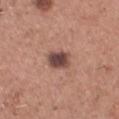This lesion was catalogued during total-body skin photography and was not selected for biopsy.
Located on the chest.
A male patient about 45 years old.
The total-body-photography lesion software estimated an area of roughly 6 mm², an eccentricity of roughly 0.7, and two-axis asymmetry of about 0.2. The analysis additionally found a lesion color around L≈45 a*≈20 b*≈22 in CIELAB, roughly 15 lightness units darker than nearby skin, and a normalized border contrast of about 11.5. It also reported a within-lesion color-variation index near 5/10 and a peripheral color-asymmetry measure near 1.5. It also reported an automated nevus-likeness rating near 50 out of 100 and a detector confidence of about 100 out of 100 that the crop contains a lesion.
This image is a 15 mm lesion crop taken from a total-body photograph.
About 3 mm across.